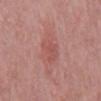Impression:
This lesion was catalogued during total-body skin photography and was not selected for biopsy.
Acquisition and patient details:
A 15 mm crop from a total-body photograph taken for skin-cancer surveillance. On the mid back. Automated tile analysis of the lesion measured a footprint of about 7 mm² and an eccentricity of roughly 0.8. The analysis additionally found a lesion color around L≈53 a*≈27 b*≈25 in CIELAB, about 6 CIELAB-L* units darker than the surrounding skin, and a normalized border contrast of about 4.5. The analysis additionally found a classifier nevus-likeness of about 0/100 and a detector confidence of about 100 out of 100 that the crop contains a lesion. About 3.5 mm across. A male subject roughly 50 years of age.